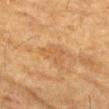Q: Was a biopsy performed?
A: no biopsy performed (imaged during a skin exam)
Q: Patient demographics?
A: male, aged approximately 85 or older
Q: What is the imaging modality?
A: total-body-photography crop, ~15 mm field of view
Q: What is the anatomic site?
A: the left forearm
Q: What lighting was used for the tile?
A: cross-polarized illumination
Q: How large is the lesion?
A: about 4.5 mm
Q: Automated lesion metrics?
A: an area of roughly 6.5 mm²; a mean CIELAB color near L≈51 a*≈19 b*≈36, about 6 CIELAB-L* units darker than the surrounding skin, and a lesion-to-skin contrast of about 4.5 (normalized; higher = more distinct); an automated nevus-likeness rating near 0 out of 100 and a lesion-detection confidence of about 95/100